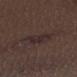Impression:
This lesion was catalogued during total-body skin photography and was not selected for biopsy.
Clinical summary:
Imaged with white-light lighting. The lesion-visualizer software estimated a shape eccentricity near 0.6 and a shape-asymmetry score of about 0.4 (0 = symmetric). The software also gave border irregularity of about 5 on a 0–10 scale and a peripheral color-asymmetry measure near 0.5. A male subject roughly 70 years of age. The lesion is located on the arm. The recorded lesion diameter is about 3.5 mm. A region of skin cropped from a whole-body photographic capture, roughly 15 mm wide.